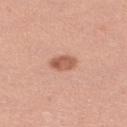<lesion>
  <biopsy_status>not biopsied; imaged during a skin examination</biopsy_status>
  <lesion_size>
    <long_diameter_mm_approx>3.0</long_diameter_mm_approx>
  </lesion_size>
  <patient>
    <sex>female</sex>
    <age_approx>35</age_approx>
  </patient>
  <site>right lower leg</site>
  <automated_metrics>
    <cielab_L>58</cielab_L>
    <cielab_a>25</cielab_a>
    <cielab_b>31</cielab_b>
    <vs_skin_darker_L>12.0</vs_skin_darker_L>
    <vs_skin_contrast_norm>8.0</vs_skin_contrast_norm>
  </automated_metrics>
  <image>
    <source>total-body photography crop</source>
    <field_of_view_mm>15</field_of_view_mm>
  </image>
  <lighting>white-light</lighting>
</lesion>